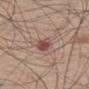Findings:
• workup · imaged on a skin check; not biopsied
• image · ~15 mm crop, total-body skin-cancer survey
• size · about 3 mm
• subject · male, aged approximately 60
• lighting · white-light illumination
• location · the left thigh